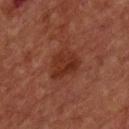No biopsy was performed on this lesion — it was imaged during a full skin examination and was not determined to be concerning.
A 15 mm close-up extracted from a 3D total-body photography capture.
Located on the chest.
A male patient aged around 65.